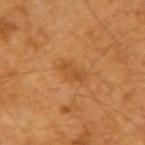Case summary:
- notes: imaged on a skin check; not biopsied
- subject: male, aged 58 to 62
- image: ~15 mm crop, total-body skin-cancer survey
- TBP lesion metrics: a footprint of about 5 mm², an eccentricity of roughly 0.8, and a symmetry-axis asymmetry near 0.3; roughly 7 lightness units darker than nearby skin and a normalized border contrast of about 5
- site: the left forearm
- illumination: cross-polarized illumination